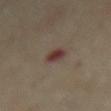<lesion>
<biopsy_status>not biopsied; imaged during a skin examination</biopsy_status>
<site>mid back</site>
<image>
  <source>total-body photography crop</source>
  <field_of_view_mm>15</field_of_view_mm>
</image>
<patient>
  <sex>female</sex>
  <age_approx>60</age_approx>
</patient>
<lesion_size>
  <long_diameter_mm_approx>2.5</long_diameter_mm_approx>
</lesion_size>
</lesion>